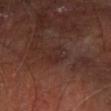{
  "site": "left forearm",
  "image": {
    "source": "total-body photography crop",
    "field_of_view_mm": 15
  },
  "lesion_size": {
    "long_diameter_mm_approx": 2.5
  },
  "patient": {
    "sex": "male",
    "age_approx": 65
  },
  "lighting": "cross-polarized",
  "automated_metrics": {
    "area_mm2_approx": 2.5,
    "border_irregularity_0_10": 5.5,
    "peripheral_color_asymmetry": 0.0
  }
}